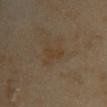<record>
  <biopsy_status>not biopsied; imaged during a skin examination</biopsy_status>
  <patient>
    <sex>female</sex>
    <age_approx>35</age_approx>
  </patient>
  <lighting>cross-polarized</lighting>
  <image>
    <source>total-body photography crop</source>
    <field_of_view_mm>15</field_of_view_mm>
  </image>
  <site>front of the torso</site>
  <automated_metrics>
    <area_mm2_approx>3.0</area_mm2_approx>
    <eccentricity>0.95</eccentricity>
    <shape_asymmetry>0.45</shape_asymmetry>
    <cielab_L>36</cielab_L>
    <cielab_a>12</cielab_a>
    <cielab_b>27</cielab_b>
    <vs_skin_darker_L>4.0</vs_skin_darker_L>
    <vs_skin_contrast_norm>5.5</vs_skin_contrast_norm>
    <color_variation_0_10>0.0</color_variation_0_10>
    <peripheral_color_asymmetry>0.0</peripheral_color_asymmetry>
    <nevus_likeness_0_100>0</nevus_likeness_0_100>
    <lesion_detection_confidence_0_100>100</lesion_detection_confidence_0_100>
  </automated_metrics>
  <lesion_size>
    <long_diameter_mm_approx>3.0</long_diameter_mm_approx>
  </lesion_size>
</record>